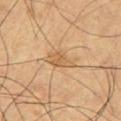biopsy status = catalogued during a skin exam; not biopsied | patient = male, aged 63 to 67 | illumination = cross-polarized illumination | image source = 15 mm crop, total-body photography | lesion size = ≈3.5 mm | site = the left thigh.